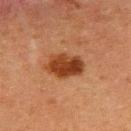No biopsy was performed on this lesion — it was imaged during a full skin examination and was not determined to be concerning.
An algorithmic analysis of the crop reported an area of roughly 11 mm², an outline eccentricity of about 0.75 (0 = round, 1 = elongated), and two-axis asymmetry of about 0.2. The software also gave a lesion color around L≈35 a*≈24 b*≈33 in CIELAB. It also reported a border-irregularity rating of about 2/10 and a color-variation rating of about 4.5/10. The analysis additionally found a nevus-likeness score of about 100/100 and a detector confidence of about 100 out of 100 that the crop contains a lesion.
A female patient aged 48–52.
This image is a 15 mm lesion crop taken from a total-body photograph.
The lesion is located on the upper back.
Imaged with cross-polarized lighting.
About 4.5 mm across.